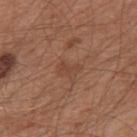No biopsy was performed on this lesion — it was imaged during a full skin examination and was not determined to be concerning.